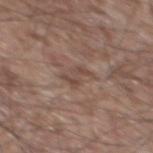{
  "biopsy_status": "not biopsied; imaged during a skin examination",
  "patient": {
    "sex": "male",
    "age_approx": 55
  },
  "automated_metrics": {
    "area_mm2_approx": 4.0,
    "eccentricity": 0.75,
    "shape_asymmetry": 0.5,
    "cielab_L": 46,
    "cielab_a": 17,
    "cielab_b": 24,
    "vs_skin_darker_L": 7.0,
    "vs_skin_contrast_norm": 5.5,
    "border_irregularity_0_10": 5.5,
    "color_variation_0_10": 2.5,
    "peripheral_color_asymmetry": 0.5,
    "nevus_likeness_0_100": 0
  },
  "lighting": "white-light",
  "site": "mid back",
  "image": {
    "source": "total-body photography crop",
    "field_of_view_mm": 15
  }
}